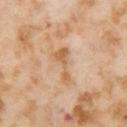Impression:
The lesion was tiled from a total-body skin photograph and was not biopsied.
Acquisition and patient details:
From the left thigh. A lesion tile, about 15 mm wide, cut from a 3D total-body photograph. Imaged with cross-polarized lighting. A female patient, roughly 55 years of age.